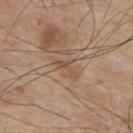This lesion was catalogued during total-body skin photography and was not selected for biopsy. On the mid back. A roughly 15 mm field-of-view crop from a total-body skin photograph. A male patient, aged 73 to 77.